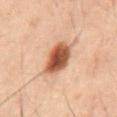Q: Was this lesion biopsied?
A: imaged on a skin check; not biopsied
Q: Where on the body is the lesion?
A: the abdomen
Q: Who is the patient?
A: male, in their 60s
Q: How large is the lesion?
A: ~4 mm (longest diameter)
Q: Automated lesion metrics?
A: a mean CIELAB color near L≈40 a*≈20 b*≈27, about 16 CIELAB-L* units darker than the surrounding skin, and a lesion-to-skin contrast of about 12 (normalized; higher = more distinct); an automated nevus-likeness rating near 100 out of 100 and a detector confidence of about 100 out of 100 that the crop contains a lesion
Q: How was this image acquired?
A: ~15 mm crop, total-body skin-cancer survey
Q: What lighting was used for the tile?
A: cross-polarized illumination